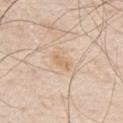Imaged during a routine full-body skin examination; the lesion was not biopsied and no histopathology is available. The total-body-photography lesion software estimated a border-irregularity rating of about 3/10, a color-variation rating of about 0/10, and a peripheral color-asymmetry measure near 0. The software also gave an automated nevus-likeness rating near 5 out of 100 and a detector confidence of about 100 out of 100 that the crop contains a lesion. Located on the chest. A 15 mm crop from a total-body photograph taken for skin-cancer surveillance. The subject is a male aged 78 to 82.